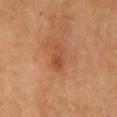Case summary:
– image · total-body-photography crop, ~15 mm field of view
– patient · female, aged around 70
– illumination · cross-polarized illumination
– size · about 3.5 mm
– site · the right upper arm